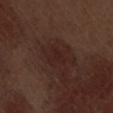Assessment: The lesion was photographed on a routine skin check and not biopsied; there is no pathology result. Clinical summary: A lesion tile, about 15 mm wide, cut from a 3D total-body photograph. The lesion is located on the back. Automated image analysis of the tile measured a shape eccentricity near 0.6 and a symmetry-axis asymmetry near 0.5. And it measured a lesion–skin lightness drop of about 4 and a normalized border contrast of about 5. And it measured border irregularity of about 5 on a 0–10 scale and peripheral color asymmetry of about 0.5. The software also gave a classifier nevus-likeness of about 0/100 and lesion-presence confidence of about 100/100. A male subject about 70 years old. The tile uses white-light illumination.